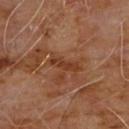Notes:
• biopsy status · imaged on a skin check; not biopsied
• imaging modality · ~15 mm tile from a whole-body skin photo
• lighting · cross-polarized illumination
• body site · the chest
• lesion diameter · ≈3.5 mm
• automated metrics · border irregularity of about 3.5 on a 0–10 scale, a within-lesion color-variation index near 0.5/10, and peripheral color asymmetry of about 0.5
• patient · male, aged 58–62